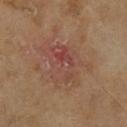Case summary:
• workup — imaged on a skin check; not biopsied
• image source — total-body-photography crop, ~15 mm field of view
• lesion diameter — about 6.5 mm
• site — the right lower leg
• patient — male, aged 63–67
• tile lighting — cross-polarized